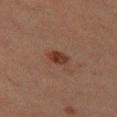Acquisition and patient details: This is a cross-polarized tile. A roughly 15 mm field-of-view crop from a total-body skin photograph. The recorded lesion diameter is about 2.5 mm. The lesion is located on the left thigh. A female subject, aged 38 to 42.